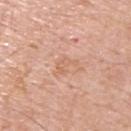biopsy status: no biopsy performed (imaged during a skin exam)
lesion size: ~2.5 mm (longest diameter)
lighting: white-light
subject: male, in their 60s
body site: the upper back
acquisition: 15 mm crop, total-body photography